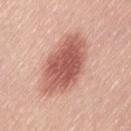Case summary:
- workup · no biopsy performed (imaged during a skin exam)
- lighting · white-light illumination
- image · 15 mm crop, total-body photography
- location · the left thigh
- automated metrics · border irregularity of about 2 on a 0–10 scale, internal color variation of about 4.5 on a 0–10 scale, and a peripheral color-asymmetry measure near 1.5; lesion-presence confidence of about 100/100
- lesion diameter · ≈7.5 mm
- patient · female, in their mid- to late 60s